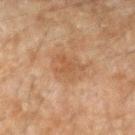workup: no biopsy performed (imaged during a skin exam)
automated lesion analysis: a footprint of about 10 mm²; an average lesion color of about L≈44 a*≈17 b*≈30 (CIELAB), roughly 6 lightness units darker than nearby skin, and a normalized lesion–skin contrast near 5
anatomic site: the left forearm
patient: male, in their mid-40s
imaging modality: ~15 mm tile from a whole-body skin photo
tile lighting: cross-polarized
diameter: about 4.5 mm